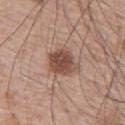This lesion was catalogued during total-body skin photography and was not selected for biopsy. A male subject, aged 63 to 67. Located on the upper back. This is a white-light tile. A lesion tile, about 15 mm wide, cut from a 3D total-body photograph. The total-body-photography lesion software estimated an area of roughly 9 mm², an eccentricity of roughly 0.4, and two-axis asymmetry of about 0.25. Longest diameter approximately 3.5 mm.